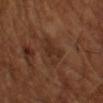biopsy status: total-body-photography surveillance lesion; no biopsy
subject: male, roughly 65 years of age
acquisition: ~15 mm tile from a whole-body skin photo
size: about 3.5 mm
lighting: cross-polarized illumination
image-analysis metrics: a footprint of about 8 mm², an eccentricity of roughly 0.65, and two-axis asymmetry of about 0.3; a lesion color around L≈29 a*≈18 b*≈25 in CIELAB, about 6 CIELAB-L* units darker than the surrounding skin, and a normalized lesion–skin contrast near 6; a border-irregularity rating of about 3.5/10, a within-lesion color-variation index near 3/10, and radial color variation of about 1